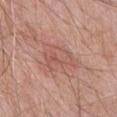Automated image analysis of the tile measured a lesion area of about 10 mm², a shape eccentricity near 0.8, and two-axis asymmetry of about 0.45. And it measured a border-irregularity rating of about 6/10, a within-lesion color-variation index near 5.5/10, and radial color variation of about 2.
A male subject, in their mid- to late 60s.
Imaged with white-light lighting.
The recorded lesion diameter is about 4.5 mm.
A region of skin cropped from a whole-body photographic capture, roughly 15 mm wide.
Located on the front of the torso.